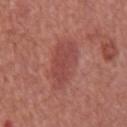biopsy status: imaged on a skin check; not biopsied
automated metrics: a lesion area of about 13 mm², an eccentricity of roughly 0.85, and a shape-asymmetry score of about 0.3 (0 = symmetric); border irregularity of about 3.5 on a 0–10 scale, a color-variation rating of about 2/10, and a peripheral color-asymmetry measure near 0.5; lesion-presence confidence of about 100/100
site: the back
patient: male, aged around 65
imaging modality: 15 mm crop, total-body photography
lighting: white-light illumination
size: ~5.5 mm (longest diameter)